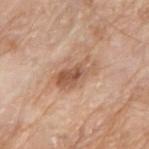{
  "biopsy_status": "not biopsied; imaged during a skin examination",
  "patient": {
    "sex": "male",
    "age_approx": 80
  },
  "image": {
    "source": "total-body photography crop",
    "field_of_view_mm": 15
  },
  "site": "arm"
}